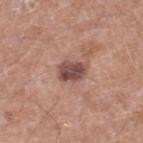This lesion was catalogued during total-body skin photography and was not selected for biopsy. The patient is a male aged 58 to 62. The lesion is located on the left lower leg. Cropped from a whole-body photographic skin survey; the tile spans about 15 mm. Automated tile analysis of the lesion measured a lesion color around L≈47 a*≈20 b*≈23 in CIELAB, a lesion–skin lightness drop of about 14, and a normalized lesion–skin contrast near 10.5. It also reported a nevus-likeness score of about 20/100. The recorded lesion diameter is about 3 mm.